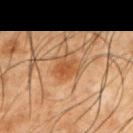The lesion was photographed on a routine skin check and not biopsied; there is no pathology result. A roughly 15 mm field-of-view crop from a total-body skin photograph. On the left upper arm. A male subject, aged 48–52.